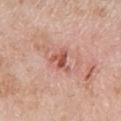Findings:
- biopsy status · total-body-photography surveillance lesion; no biopsy
- diameter · ~3 mm (longest diameter)
- subject · female, about 70 years old
- site · the left lower leg
- imaging modality · 15 mm crop, total-body photography
- tile lighting · white-light